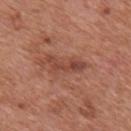Clinical impression:
Recorded during total-body skin imaging; not selected for excision or biopsy.
Clinical summary:
From the mid back. The recorded lesion diameter is about 4.5 mm. The tile uses white-light illumination. A male subject, aged 68–72. An algorithmic analysis of the crop reported a lesion area of about 6 mm² and a shape-asymmetry score of about 0.4 (0 = symmetric). And it measured a nevus-likeness score of about 5/100 and a detector confidence of about 100 out of 100 that the crop contains a lesion. A 15 mm close-up extracted from a 3D total-body photography capture.